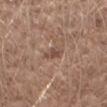notes = imaged on a skin check; not biopsied
site = the arm
image source = ~15 mm tile from a whole-body skin photo
subject = male, roughly 60 years of age